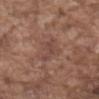| field | value |
|---|---|
| image source | total-body-photography crop, ~15 mm field of view |
| patient | male, approximately 75 years of age |
| body site | the abdomen |